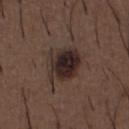Q: Illumination type?
A: white-light illumination
Q: Automated lesion metrics?
A: a lesion color around L≈26 a*≈13 b*≈17 in CIELAB and about 11 CIELAB-L* units darker than the surrounding skin
Q: Who is the patient?
A: male, roughly 50 years of age
Q: What is the imaging modality?
A: ~15 mm tile from a whole-body skin photo
Q: Where on the body is the lesion?
A: the front of the torso
Q: How large is the lesion?
A: ~4.5 mm (longest diameter)